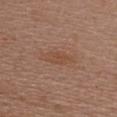This lesion was catalogued during total-body skin photography and was not selected for biopsy.
This is a white-light tile.
On the chest.
A female patient in their 60s.
A lesion tile, about 15 mm wide, cut from a 3D total-body photograph.
The lesion's longest dimension is about 4 mm.